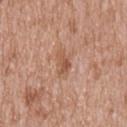  biopsy_status: not biopsied; imaged during a skin examination
  image:
    source: total-body photography crop
    field_of_view_mm: 15
  site: mid back
  patient:
    sex: male
    age_approx: 65